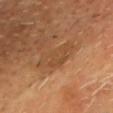Clinical impression:
Recorded during total-body skin imaging; not selected for excision or biopsy.
Background:
Approximately 5.5 mm at its widest. The lesion is located on the chest. A close-up tile cropped from a whole-body skin photograph, about 15 mm across. This is a cross-polarized tile. Automated image analysis of the tile measured a lesion color around L≈48 a*≈21 b*≈37 in CIELAB, about 6 CIELAB-L* units darker than the surrounding skin, and a normalized lesion–skin contrast near 5. The patient is a male about 65 years old.